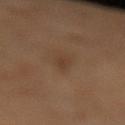No biopsy was performed on this lesion — it was imaged during a full skin examination and was not determined to be concerning. From the left lower leg. A female subject in their 50s. Measured at roughly 2.5 mm in maximum diameter. A 15 mm crop from a total-body photograph taken for skin-cancer surveillance.